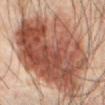Assessment:
Imaged during a routine full-body skin examination; the lesion was not biopsied and no histopathology is available.
Image and clinical context:
Imaged with cross-polarized lighting. Longest diameter approximately 11 mm. The subject is a male about 65 years old. A 15 mm close-up tile from a total-body photography series done for melanoma screening. Located on the abdomen.